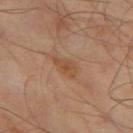Context: Cropped from a whole-body photographic skin survey; the tile spans about 15 mm. Measured at roughly 3 mm in maximum diameter. A male patient in their mid- to late 40s. The lesion is located on the left thigh. Captured under cross-polarized illumination.